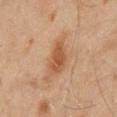workup — no biopsy performed (imaged during a skin exam) | patient — male, aged 43–47 | tile lighting — cross-polarized illumination | site — the back | imaging modality — ~15 mm tile from a whole-body skin photo.